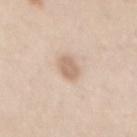Impression:
This lesion was catalogued during total-body skin photography and was not selected for biopsy.
Context:
A female subject, approximately 40 years of age. A 15 mm crop from a total-body photograph taken for skin-cancer surveillance. The lesion is on the mid back.